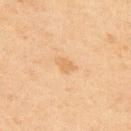Recorded during total-body skin imaging; not selected for excision or biopsy. Located on the upper back. This image is a 15 mm lesion crop taken from a total-body photograph. The subject is a male aged 43 to 47.